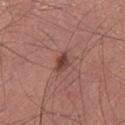Recorded during total-body skin imaging; not selected for excision or biopsy. Captured under white-light illumination. Approximately 2.5 mm at its widest. A 15 mm close-up extracted from a 3D total-body photography capture. On the left lower leg. A male patient, aged approximately 60.